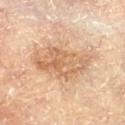patient: female, aged 78–82 | site: the right leg | imaging modality: 15 mm crop, total-body photography | automated metrics: an outline eccentricity of about 0.8 (0 = round, 1 = elongated) and two-axis asymmetry of about 0.3; a border-irregularity rating of about 4/10, internal color variation of about 5 on a 0–10 scale, and peripheral color asymmetry of about 1.5; a nevus-likeness score of about 5/100 and lesion-presence confidence of about 100/100 | lighting: cross-polarized.